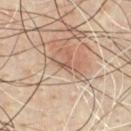Recorded during total-body skin imaging; not selected for excision or biopsy. A close-up tile cropped from a whole-body skin photograph, about 15 mm across. On the chest. The tile uses cross-polarized illumination. The recorded lesion diameter is about 1.5 mm. A male patient, roughly 40 years of age. The total-body-photography lesion software estimated a mean CIELAB color near L≈55 a*≈20 b*≈29 and about 9 CIELAB-L* units darker than the surrounding skin. The software also gave a classifier nevus-likeness of about 0/100 and a lesion-detection confidence of about 0/100.